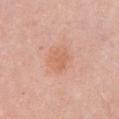anatomic site = the chest | lesion size = about 3.5 mm | tile lighting = white-light illumination | TBP lesion metrics = an eccentricity of roughly 0.5; an average lesion color of about L≈64 a*≈24 b*≈33 (CIELAB), a lesion–skin lightness drop of about 6, and a normalized lesion–skin contrast near 5; a classifier nevus-likeness of about 10/100 and a lesion-detection confidence of about 100/100 | subject = female, aged approximately 30 | image = total-body-photography crop, ~15 mm field of view.